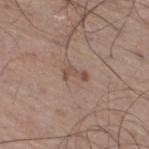Assessment:
Imaged during a routine full-body skin examination; the lesion was not biopsied and no histopathology is available.